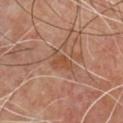– biopsy status · no biopsy performed (imaged during a skin exam)
– image · 15 mm crop, total-body photography
– anatomic site · the front of the torso
– illumination · cross-polarized
– subject · male, aged around 70
– image-analysis metrics · a mean CIELAB color near L≈47 a*≈21 b*≈31, a lesion–skin lightness drop of about 7, and a normalized lesion–skin contrast near 6; a lesion-detection confidence of about 100/100
– diameter · ≈3.5 mm